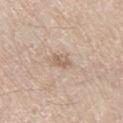notes = imaged on a skin check; not biopsied
anatomic site = the leg
subject = male, aged approximately 80
acquisition = ~15 mm crop, total-body skin-cancer survey
illumination = white-light illumination
diameter = about 2.5 mm